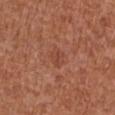{"biopsy_status": "not biopsied; imaged during a skin examination", "image": {"source": "total-body photography crop", "field_of_view_mm": 15}, "patient": {"sex": "female", "age_approx": 65}, "site": "left arm"}